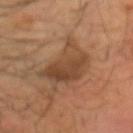This lesion was catalogued during total-body skin photography and was not selected for biopsy.
Located on the head or neck.
The subject is a male aged 53–57.
Imaged with cross-polarized lighting.
Cropped from a whole-body photographic skin survey; the tile spans about 15 mm.
The lesion's longest dimension is about 6 mm.
The total-body-photography lesion software estimated an area of roughly 19 mm², a shape eccentricity near 0.6, and a shape-asymmetry score of about 0.35 (0 = symmetric). The analysis additionally found border irregularity of about 5 on a 0–10 scale, internal color variation of about 4.5 on a 0–10 scale, and peripheral color asymmetry of about 1.5. And it measured a classifier nevus-likeness of about 55/100 and a lesion-detection confidence of about 100/100.